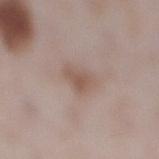A female patient, in their 30s. A close-up tile cropped from a whole-body skin photograph, about 15 mm across. From the right lower leg. Imaged with white-light lighting. Automated tile analysis of the lesion measured a mean CIELAB color near L≈55 a*≈16 b*≈24, about 8 CIELAB-L* units darker than the surrounding skin, and a normalized lesion–skin contrast near 6.